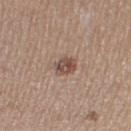Acquisition and patient details: On the right thigh. The subject is a female in their 40s. The lesion-visualizer software estimated a symmetry-axis asymmetry near 0.25. The software also gave a lesion color around L≈49 a*≈17 b*≈24 in CIELAB, about 11 CIELAB-L* units darker than the surrounding skin, and a lesion-to-skin contrast of about 8 (normalized; higher = more distinct). And it measured a border-irregularity rating of about 2.5/10, a within-lesion color-variation index near 4/10, and radial color variation of about 1.5. The software also gave a classifier nevus-likeness of about 45/100. Measured at roughly 2.5 mm in maximum diameter. A region of skin cropped from a whole-body photographic capture, roughly 15 mm wide.